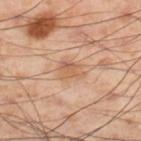Imaged during a routine full-body skin examination; the lesion was not biopsied and no histopathology is available.
The patient is a male aged 53 to 57.
About 3 mm across.
The lesion is located on the leg.
The total-body-photography lesion software estimated a footprint of about 5.5 mm² and an eccentricity of roughly 0.65. It also reported a border-irregularity index near 3.5/10, a color-variation rating of about 4.5/10, and radial color variation of about 1.5. The analysis additionally found a classifier nevus-likeness of about 0/100 and a detector confidence of about 100 out of 100 that the crop contains a lesion.
A region of skin cropped from a whole-body photographic capture, roughly 15 mm wide.
Captured under cross-polarized illumination.